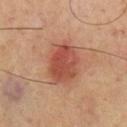notes = no biopsy performed (imaged during a skin exam) | lesion diameter = ~5 mm (longest diameter) | anatomic site = the chest | imaging modality = total-body-photography crop, ~15 mm field of view | patient = male, roughly 70 years of age.